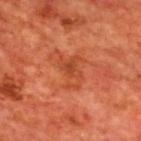The recorded lesion diameter is about 4 mm. This is a cross-polarized tile. The subject is a male aged approximately 70. On the upper back. A close-up tile cropped from a whole-body skin photograph, about 15 mm across.